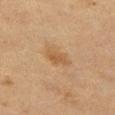Q: Is there a histopathology result?
A: no biopsy performed (imaged during a skin exam)
Q: Lesion size?
A: ~3.5 mm (longest diameter)
Q: What did automated image analysis measure?
A: border irregularity of about 3 on a 0–10 scale, a color-variation rating of about 3/10, and radial color variation of about 1; a classifier nevus-likeness of about 50/100 and a detector confidence of about 100 out of 100 that the crop contains a lesion
Q: What is the imaging modality?
A: ~15 mm tile from a whole-body skin photo
Q: How was the tile lit?
A: cross-polarized
Q: Lesion location?
A: the left thigh
Q: Patient demographics?
A: female, approximately 40 years of age